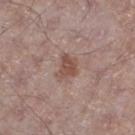Impression: Imaged during a routine full-body skin examination; the lesion was not biopsied and no histopathology is available. Image and clinical context: The subject is a male roughly 65 years of age. The lesion is located on the leg. This is a white-light tile. This image is a 15 mm lesion crop taken from a total-body photograph. The lesion's longest dimension is about 3 mm. Automated tile analysis of the lesion measured a mean CIELAB color near L≈50 a*≈19 b*≈24, roughly 9 lightness units darker than nearby skin, and a normalized lesion–skin contrast near 7. It also reported lesion-presence confidence of about 100/100.